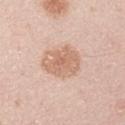Clinical impression:
No biopsy was performed on this lesion — it was imaged during a full skin examination and was not determined to be concerning.
Image and clinical context:
A roughly 15 mm field-of-view crop from a total-body skin photograph. From the right upper arm. Imaged with white-light lighting. Measured at roughly 5 mm in maximum diameter. A male subject roughly 45 years of age.